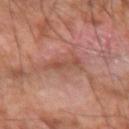Assessment: Part of a total-body skin-imaging series; this lesion was reviewed on a skin check and was not flagged for biopsy. Background: Located on the left forearm. Approximately 4 mm at its widest. The patient is a male approximately 60 years of age. Cropped from a whole-body photographic skin survey; the tile spans about 15 mm.